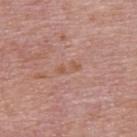Notes:
- biopsy status · no biopsy performed (imaged during a skin exam)
- image · ~15 mm tile from a whole-body skin photo
- size · ~3 mm (longest diameter)
- patient · male, aged 73 to 77
- site · the upper back
- lighting · white-light